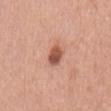| feature | finding |
|---|---|
| notes | total-body-photography surveillance lesion; no biopsy |
| lesion size | ~3 mm (longest diameter) |
| image | ~15 mm tile from a whole-body skin photo |
| patient | male, about 70 years old |
| image-analysis metrics | a footprint of about 5 mm² and a symmetry-axis asymmetry near 0.25; border irregularity of about 2.5 on a 0–10 scale and a within-lesion color-variation index near 3.5/10; a nevus-likeness score of about 95/100 and a detector confidence of about 100 out of 100 that the crop contains a lesion |
| illumination | white-light |
| body site | the mid back |